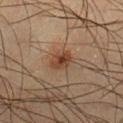A 15 mm crop from a total-body photograph taken for skin-cancer surveillance. Captured under cross-polarized illumination. A male subject, about 35 years old. The lesion's longest dimension is about 2.5 mm. On the leg.